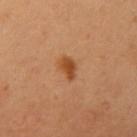Clinical impression: Captured during whole-body skin photography for melanoma surveillance; the lesion was not biopsied. Image and clinical context: On the right upper arm. This image is a 15 mm lesion crop taken from a total-body photograph. The patient is a female aged 23 to 27.